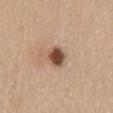follow-up: total-body-photography surveillance lesion; no biopsy
patient: female, aged 63 to 67
tile lighting: white-light
lesion size: ~3 mm (longest diameter)
image source: ~15 mm crop, total-body skin-cancer survey
site: the lower back
TBP lesion metrics: a footprint of about 6 mm², an outline eccentricity of about 0.55 (0 = round, 1 = elongated), and a symmetry-axis asymmetry near 0.2